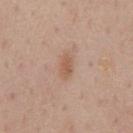| field | value |
|---|---|
| follow-up | imaged on a skin check; not biopsied |
| anatomic site | the front of the torso |
| automated metrics | a lesion area of about 3.5 mm²; a mean CIELAB color near L≈57 a*≈20 b*≈31, a lesion–skin lightness drop of about 8, and a normalized lesion–skin contrast near 6.5 |
| lighting | white-light |
| subject | male, aged approximately 60 |
| acquisition | ~15 mm tile from a whole-body skin photo |